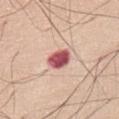biopsy status — no biopsy performed (imaged during a skin exam)
automated metrics — a footprint of about 6 mm², an outline eccentricity of about 0.65 (0 = round, 1 = elongated), and two-axis asymmetry of about 0.15; a nevus-likeness score of about 0/100 and lesion-presence confidence of about 100/100
patient — male, aged approximately 60
image — ~15 mm crop, total-body skin-cancer survey
size — ≈3 mm
location — the abdomen